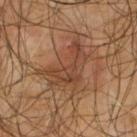The lesion was tiled from a total-body skin photograph and was not biopsied.
Cropped from a total-body skin-imaging series; the visible field is about 15 mm.
On the upper back.
The recorded lesion diameter is about 6 mm.
Automated tile analysis of the lesion measured a lesion area of about 16 mm², an eccentricity of roughly 0.75, and two-axis asymmetry of about 0.6. And it measured border irregularity of about 8 on a 0–10 scale and a peripheral color-asymmetry measure near 1.
The subject is a male approximately 65 years of age.